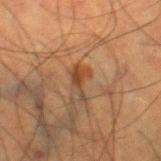Assessment: This lesion was catalogued during total-body skin photography and was not selected for biopsy.